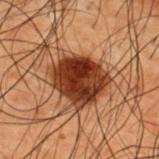workup: total-body-photography surveillance lesion; no biopsy | diameter: ~5.5 mm (longest diameter) | patient: male, roughly 50 years of age | tile lighting: cross-polarized | automated metrics: a mean CIELAB color near L≈27 a*≈21 b*≈27 and a lesion-to-skin contrast of about 14.5 (normalized; higher = more distinct); border irregularity of about 2 on a 0–10 scale | location: the upper back | image source: total-body-photography crop, ~15 mm field of view.